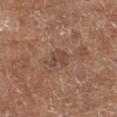  patient:
    sex: female
    age_approx: 75
  site: right forearm
  image:
    source: total-body photography crop
    field_of_view_mm: 15